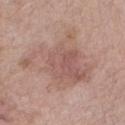{
  "biopsy_status": "not biopsied; imaged during a skin examination",
  "lesion_size": {
    "long_diameter_mm_approx": 7.5
  },
  "automated_metrics": {
    "cielab_L": 56,
    "cielab_a": 19,
    "cielab_b": 24,
    "vs_skin_darker_L": 8.0,
    "vs_skin_contrast_norm": 5.5,
    "border_irregularity_0_10": 7.5,
    "color_variation_0_10": 5.0,
    "peripheral_color_asymmetry": 1.5,
    "nevus_likeness_0_100": 0,
    "lesion_detection_confidence_0_100": 100
  },
  "image": {
    "source": "total-body photography crop",
    "field_of_view_mm": 15
  },
  "lighting": "white-light",
  "site": "right forearm",
  "patient": {
    "sex": "male",
    "age_approx": 60
  }
}